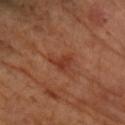Q: Is there a histopathology result?
A: imaged on a skin check; not biopsied
Q: What did automated image analysis measure?
A: a footprint of about 4 mm² and two-axis asymmetry of about 0.5
Q: What is the lesion's diameter?
A: ≈3 mm
Q: Lesion location?
A: the right forearm
Q: What is the imaging modality?
A: ~15 mm tile from a whole-body skin photo
Q: What are the patient's age and sex?
A: female
Q: How was the tile lit?
A: cross-polarized illumination